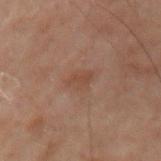biopsy_status: not biopsied; imaged during a skin examination
patient:
  sex: male
  age_approx: 70
lesion_size:
  long_diameter_mm_approx: 2.5
image:
  source: total-body photography crop
  field_of_view_mm: 15
site: arm
automated_metrics:
  cielab_L: 34
  cielab_a: 15
  cielab_b: 22
  vs_skin_darker_L: 4.0
  vs_skin_contrast_norm: 5.0
  color_variation_0_10: 1.5
  peripheral_color_asymmetry: 0.5
  nevus_likeness_0_100: 0
  lesion_detection_confidence_0_100: 100